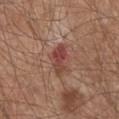workup: no biopsy performed (imaged during a skin exam) | location: the chest | lesion diameter: ≈4 mm | image source: ~15 mm crop, total-body skin-cancer survey | TBP lesion metrics: an eccentricity of roughly 0.85 and a shape-asymmetry score of about 0.25 (0 = symmetric); roughly 10 lightness units darker than nearby skin; lesion-presence confidence of about 100/100 | lighting: white-light | patient: male, approximately 65 years of age.